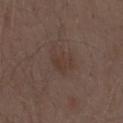The lesion was tiled from a total-body skin photograph and was not biopsied. The tile uses white-light illumination. The lesion-visualizer software estimated a mean CIELAB color near L≈36 a*≈14 b*≈22, a lesion–skin lightness drop of about 5, and a normalized lesion–skin contrast near 5. And it measured a border-irregularity index near 5.5/10, a color-variation rating of about 2/10, and radial color variation of about 0.5. A male subject aged around 70. On the chest. A close-up tile cropped from a whole-body skin photograph, about 15 mm across. Measured at roughly 3.5 mm in maximum diameter.